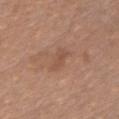{
  "biopsy_status": "not biopsied; imaged during a skin examination",
  "site": "chest",
  "patient": {
    "sex": "female",
    "age_approx": 55
  },
  "image": {
    "source": "total-body photography crop",
    "field_of_view_mm": 15
  }
}